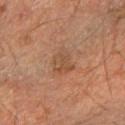Clinical impression: This lesion was catalogued during total-body skin photography and was not selected for biopsy. Image and clinical context: From the right forearm. The subject is a male approximately 65 years of age. A 15 mm close-up tile from a total-body photography series done for melanoma screening. Automated image analysis of the tile measured a lesion area of about 6.5 mm² and an eccentricity of roughly 0.75. The software also gave roughly 6 lightness units darker than nearby skin and a lesion-to-skin contrast of about 5 (normalized; higher = more distinct). It also reported a border-irregularity index near 3/10 and a within-lesion color-variation index near 3.5/10. The analysis additionally found an automated nevus-likeness rating near 0 out of 100 and a lesion-detection confidence of about 100/100. The recorded lesion diameter is about 3.5 mm.